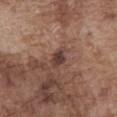Assessment:
The lesion was photographed on a routine skin check and not biopsied; there is no pathology result.
Image and clinical context:
A male subject, approximately 75 years of age. From the abdomen. Measured at roughly 3 mm in maximum diameter. A roughly 15 mm field-of-view crop from a total-body skin photograph. The lesion-visualizer software estimated an area of roughly 4 mm² and a symmetry-axis asymmetry near 0.3. And it measured a classifier nevus-likeness of about 45/100 and a detector confidence of about 100 out of 100 that the crop contains a lesion.